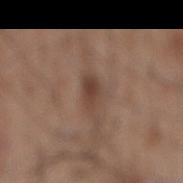Case summary:
• follow-up: total-body-photography surveillance lesion; no biopsy
• location: the mid back
• size: ≈3 mm
• imaging modality: ~15 mm crop, total-body skin-cancer survey
• illumination: white-light illumination
• subject: male, aged around 60
• image-analysis metrics: an area of roughly 4 mm², an outline eccentricity of about 0.75 (0 = round, 1 = elongated), and two-axis asymmetry of about 0.25; a border-irregularity index near 2/10, internal color variation of about 3.5 on a 0–10 scale, and peripheral color asymmetry of about 1.5; a classifier nevus-likeness of about 35/100 and lesion-presence confidence of about 100/100